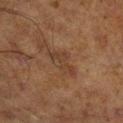Part of a total-body skin-imaging series; this lesion was reviewed on a skin check and was not flagged for biopsy.
A 15 mm close-up tile from a total-body photography series done for melanoma screening.
Captured under cross-polarized illumination.
Located on the right lower leg.
The recorded lesion diameter is about 4 mm.
A male subject, roughly 70 years of age.
An algorithmic analysis of the crop reported an area of roughly 6 mm², an outline eccentricity of about 0.85 (0 = round, 1 = elongated), and a symmetry-axis asymmetry near 0.4. And it measured an average lesion color of about L≈31 a*≈15 b*≈25 (CIELAB), roughly 6 lightness units darker than nearby skin, and a lesion-to-skin contrast of about 6 (normalized; higher = more distinct). It also reported border irregularity of about 5 on a 0–10 scale, a color-variation rating of about 2/10, and radial color variation of about 0.5.